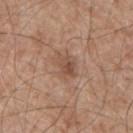{
  "biopsy_status": "not biopsied; imaged during a skin examination",
  "lighting": "white-light",
  "automated_metrics": {
    "cielab_L": 50,
    "cielab_a": 19,
    "cielab_b": 28,
    "vs_skin_darker_L": 9.0,
    "vs_skin_contrast_norm": 6.5,
    "nevus_likeness_0_100": 0,
    "lesion_detection_confidence_0_100": 100
  },
  "site": "mid back",
  "image": {
    "source": "total-body photography crop",
    "field_of_view_mm": 15
  },
  "patient": {
    "sex": "male",
    "age_approx": 55
  }
}